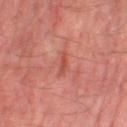Impression:
The lesion was tiled from a total-body skin photograph and was not biopsied.
Image and clinical context:
The lesion is on the right lower leg. A lesion tile, about 15 mm wide, cut from a 3D total-body photograph. Measured at roughly 2.5 mm in maximum diameter. The patient is a male in their mid- to late 50s. Imaged with cross-polarized lighting.